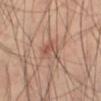| feature | finding |
|---|---|
| biopsy status | no biopsy performed (imaged during a skin exam) |
| tile lighting | cross-polarized illumination |
| anatomic site | the right thigh |
| imaging modality | ~15 mm tile from a whole-body skin photo |
| TBP lesion metrics | border irregularity of about 3 on a 0–10 scale, a within-lesion color-variation index near 5.5/10, and a peripheral color-asymmetry measure near 2; a nevus-likeness score of about 0/100 and a detector confidence of about 100 out of 100 that the crop contains a lesion |
| subject | male, roughly 60 years of age |
| lesion diameter | about 3.5 mm |